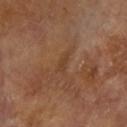Clinical impression: No biopsy was performed on this lesion — it was imaged during a full skin examination and was not determined to be concerning. Acquisition and patient details: Cropped from a total-body skin-imaging series; the visible field is about 15 mm. A female subject aged approximately 75. Imaged with cross-polarized lighting. On the left forearm. An algorithmic analysis of the crop reported a lesion area of about 2.5 mm², an eccentricity of roughly 0.9, and a shape-asymmetry score of about 0.3 (0 = symmetric). It also reported an average lesion color of about L≈40 a*≈20 b*≈32 (CIELAB) and a normalized border contrast of about 5. And it measured border irregularity of about 3.5 on a 0–10 scale and a color-variation rating of about 0/10. And it measured an automated nevus-likeness rating near 0 out of 100 and a detector confidence of about 85 out of 100 that the crop contains a lesion.